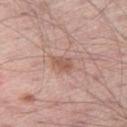No biopsy was performed on this lesion — it was imaged during a full skin examination and was not determined to be concerning. On the right thigh. The total-body-photography lesion software estimated an area of roughly 3.5 mm², an eccentricity of roughly 0.8, and a symmetry-axis asymmetry near 0.3. Captured under white-light illumination. A male subject, aged approximately 55. A close-up tile cropped from a whole-body skin photograph, about 15 mm across. The recorded lesion diameter is about 2.5 mm.